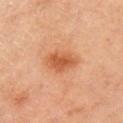Assessment: Part of a total-body skin-imaging series; this lesion was reviewed on a skin check and was not flagged for biopsy. Background: Measured at roughly 4 mm in maximum diameter. A close-up tile cropped from a whole-body skin photograph, about 15 mm across. A female subject roughly 65 years of age. This is a cross-polarized tile. Located on the right upper arm.